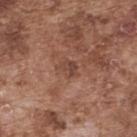biopsy_status: not biopsied; imaged during a skin examination
site: upper back
image:
  source: total-body photography crop
  field_of_view_mm: 15
lighting: white-light
patient:
  sex: male
  age_approx: 75